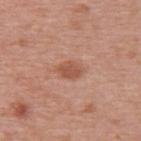Clinical impression: The lesion was tiled from a total-body skin photograph and was not biopsied. Image and clinical context: From the upper back. A female patient, aged approximately 40. Imaged with white-light lighting. Cropped from a whole-body photographic skin survey; the tile spans about 15 mm.